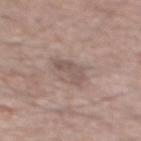Part of a total-body skin-imaging series; this lesion was reviewed on a skin check and was not flagged for biopsy.
The total-body-photography lesion software estimated a lesion area of about 5.5 mm², an eccentricity of roughly 0.85, and a shape-asymmetry score of about 0.4 (0 = symmetric). And it measured a classifier nevus-likeness of about 0/100 and a lesion-detection confidence of about 100/100.
A male patient in their mid- to late 50s.
The lesion is on the back.
Longest diameter approximately 3.5 mm.
A 15 mm crop from a total-body photograph taken for skin-cancer surveillance.